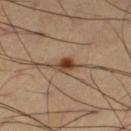Recorded during total-body skin imaging; not selected for excision or biopsy.
The lesion is located on the leg.
About 2.5 mm across.
Imaged with cross-polarized lighting.
The total-body-photography lesion software estimated a footprint of about 4 mm², an outline eccentricity of about 0.75 (0 = round, 1 = elongated), and two-axis asymmetry of about 0.35. The analysis additionally found a lesion-to-skin contrast of about 10 (normalized; higher = more distinct). It also reported a border-irregularity index near 3/10, internal color variation of about 4.5 on a 0–10 scale, and radial color variation of about 1.5.
A 15 mm crop from a total-body photograph taken for skin-cancer surveillance.
A male patient, in their mid- to late 60s.